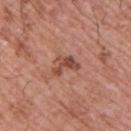The lesion was tiled from a total-body skin photograph and was not biopsied.
Captured under white-light illumination.
The subject is a male in their mid-60s.
The lesion is on the upper back.
This image is a 15 mm lesion crop taken from a total-body photograph.